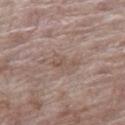Part of a total-body skin-imaging series; this lesion was reviewed on a skin check and was not flagged for biopsy. This is a white-light tile. On the right thigh. A female subject aged 83 or over. Cropped from a total-body skin-imaging series; the visible field is about 15 mm. The recorded lesion diameter is about 3 mm.